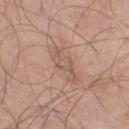Clinical impression: Captured during whole-body skin photography for melanoma surveillance; the lesion was not biopsied. Clinical summary: A 15 mm close-up extracted from a 3D total-body photography capture. The lesion is on the right thigh. A male subject aged 68–72.